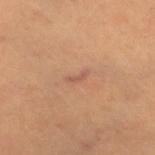Captured during whole-body skin photography for melanoma surveillance; the lesion was not biopsied.
This image is a 15 mm lesion crop taken from a total-body photograph.
About 2.5 mm across.
A female subject, approximately 45 years of age.
Captured under cross-polarized illumination.
Located on the leg.
The total-body-photography lesion software estimated a footprint of about 2 mm² and an eccentricity of roughly 0.95. And it measured a lesion color around L≈55 a*≈22 b*≈30 in CIELAB and a lesion-to-skin contrast of about 5.5 (normalized; higher = more distinct). It also reported a border-irregularity rating of about 5/10 and peripheral color asymmetry of about 0.